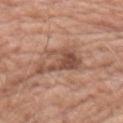The lesion was photographed on a routine skin check and not biopsied; there is no pathology result. A roughly 15 mm field-of-view crop from a total-body skin photograph. This is a white-light tile. On the right upper arm. A male patient, in their 60s.